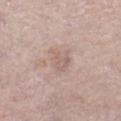Assessment:
Captured during whole-body skin photography for melanoma surveillance; the lesion was not biopsied.
Context:
Longest diameter approximately 3 mm. On the left thigh. Automated image analysis of the tile measured a classifier nevus-likeness of about 0/100 and lesion-presence confidence of about 90/100. A female patient aged around 60. A 15 mm close-up extracted from a 3D total-body photography capture.